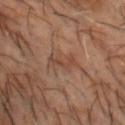Captured during whole-body skin photography for melanoma surveillance; the lesion was not biopsied. This image is a 15 mm lesion crop taken from a total-body photograph. The lesion's longest dimension is about 3.5 mm. Automated image analysis of the tile measured a color-variation rating of about 2/10 and radial color variation of about 0.5. A male subject roughly 50 years of age. The lesion is on the head or neck.